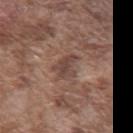diameter: ≈3.5 mm
body site: the front of the torso
TBP lesion metrics: a footprint of about 6 mm² and a symmetry-axis asymmetry near 0.4; an average lesion color of about L≈44 a*≈17 b*≈23 (CIELAB), about 9 CIELAB-L* units darker than the surrounding skin, and a lesion-to-skin contrast of about 7.5 (normalized; higher = more distinct); a border-irregularity rating of about 4.5/10 and peripheral color asymmetry of about 1
imaging modality: ~15 mm crop, total-body skin-cancer survey
subject: male, aged 73 to 77
illumination: white-light